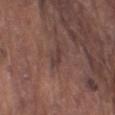notes: no biopsy performed (imaged during a skin exam); illumination: white-light; image: ~15 mm tile from a whole-body skin photo; subject: male, aged around 80; body site: the left upper arm.